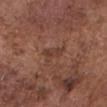{"lighting": "white-light", "lesion_size": {"long_diameter_mm_approx": 3.0}, "site": "head or neck", "patient": {"sex": "male", "age_approx": 75}, "image": {"source": "total-body photography crop", "field_of_view_mm": 15}}